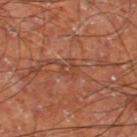Assessment:
No biopsy was performed on this lesion — it was imaged during a full skin examination and was not determined to be concerning.
Image and clinical context:
A region of skin cropped from a whole-body photographic capture, roughly 15 mm wide. The lesion is on the left thigh. A male subject approximately 60 years of age.